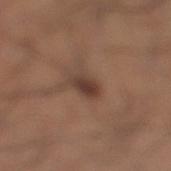<case>
  <image>
    <source>total-body photography crop</source>
    <field_of_view_mm>15</field_of_view_mm>
  </image>
  <lesion_size>
    <long_diameter_mm_approx>3.0</long_diameter_mm_approx>
  </lesion_size>
  <site>right lower leg</site>
  <patient>
    <sex>male</sex>
    <age_approx>35</age_approx>
  </patient>
  <lighting>white-light</lighting>
</case>